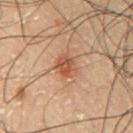Findings:
– workup · total-body-photography surveillance lesion; no biopsy
– body site · the front of the torso
– illumination · cross-polarized
– patient · male, about 50 years old
– image-analysis metrics · a nevus-likeness score of about 85/100 and a lesion-detection confidence of about 100/100
– imaging modality · ~15 mm crop, total-body skin-cancer survey